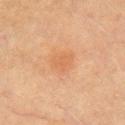Imaged during a routine full-body skin examination; the lesion was not biopsied and no histopathology is available. This is a cross-polarized tile. A lesion tile, about 15 mm wide, cut from a 3D total-body photograph. The patient is a male aged around 70. The lesion is located on the arm.